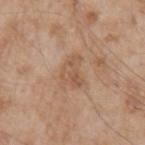follow-up: imaged on a skin check; not biopsied
subject: male, aged 63 to 67
size: about 3.5 mm
site: the left upper arm
image-analysis metrics: a border-irregularity rating of about 5/10, a color-variation rating of about 4/10, and a peripheral color-asymmetry measure near 1.5
tile lighting: white-light illumination
imaging modality: 15 mm crop, total-body photography